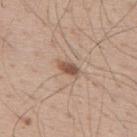Assessment:
Imaged during a routine full-body skin examination; the lesion was not biopsied and no histopathology is available.
Context:
A male subject, aged 38 to 42. On the upper back. A roughly 15 mm field-of-view crop from a total-body skin photograph.